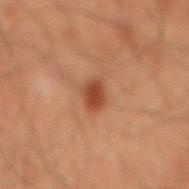Case summary:
* workup: total-body-photography surveillance lesion; no biopsy
* location: the mid back
* subject: male, in their 60s
* image: ~15 mm tile from a whole-body skin photo